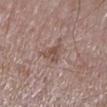Q: Is there a histopathology result?
A: no biopsy performed (imaged during a skin exam)
Q: What is the imaging modality?
A: ~15 mm crop, total-body skin-cancer survey
Q: What did automated image analysis measure?
A: a lesion area of about 5 mm², an outline eccentricity of about 0.8 (0 = round, 1 = elongated), and two-axis asymmetry of about 0.45; a lesion color around L≈50 a*≈17 b*≈24 in CIELAB and a lesion-to-skin contrast of about 6.5 (normalized; higher = more distinct); a border-irregularity index near 4.5/10, a color-variation rating of about 3/10, and peripheral color asymmetry of about 1
Q: What is the anatomic site?
A: the arm
Q: Lesion size?
A: ~3.5 mm (longest diameter)
Q: Who is the patient?
A: male, aged 63 to 67
Q: How was the tile lit?
A: white-light